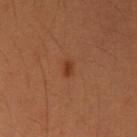workup = imaged on a skin check; not biopsied | tile lighting = cross-polarized | size = ≈1.5 mm | image source = ~15 mm tile from a whole-body skin photo | body site = the left upper arm | TBP lesion metrics = a mean CIELAB color near L≈37 a*≈24 b*≈34 and about 9 CIELAB-L* units darker than the surrounding skin; internal color variation of about 0 on a 0–10 scale and a peripheral color-asymmetry measure near 0 | subject = female, aged approximately 40.